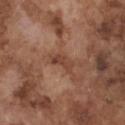Q: Is there a histopathology result?
A: imaged on a skin check; not biopsied
Q: What lighting was used for the tile?
A: white-light
Q: How large is the lesion?
A: ≈3.5 mm
Q: What is the imaging modality?
A: total-body-photography crop, ~15 mm field of view
Q: What are the patient's age and sex?
A: male, aged around 75
Q: What is the anatomic site?
A: the front of the torso